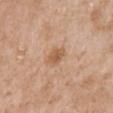Recorded during total-body skin imaging; not selected for excision or biopsy. A 15 mm close-up extracted from a 3D total-body photography capture. A male patient about 65 years old. From the chest. The lesion's longest dimension is about 2.5 mm. Captured under white-light illumination. An algorithmic analysis of the crop reported border irregularity of about 2.5 on a 0–10 scale and internal color variation of about 1.5 on a 0–10 scale. It also reported a nevus-likeness score of about 55/100 and a lesion-detection confidence of about 100/100.